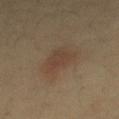Captured during whole-body skin photography for melanoma surveillance; the lesion was not biopsied.